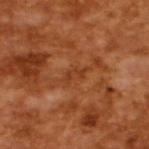{"biopsy_status": "not biopsied; imaged during a skin examination", "image": {"source": "total-body photography crop", "field_of_view_mm": 15}, "lesion_size": {"long_diameter_mm_approx": 2.5}, "lighting": "cross-polarized", "patient": {"sex": "male", "age_approx": 65}}